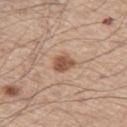{"biopsy_status": "not biopsied; imaged during a skin examination", "automated_metrics": {"nevus_likeness_0_100": 90, "lesion_detection_confidence_0_100": 100}, "patient": {"sex": "male", "age_approx": 60}, "image": {"source": "total-body photography crop", "field_of_view_mm": 15}, "site": "left thigh", "lesion_size": {"long_diameter_mm_approx": 3.0}, "lighting": "white-light"}